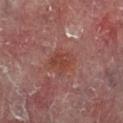Background:
A male subject roughly 60 years of age. From the right lower leg. This image is a 15 mm lesion crop taken from a total-body photograph.